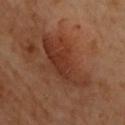The lesion was tiled from a total-body skin photograph and was not biopsied.
A 15 mm close-up extracted from a 3D total-body photography capture.
The patient is a female roughly 60 years of age.
Longest diameter approximately 6.5 mm.
Located on the upper back.
Captured under cross-polarized illumination.